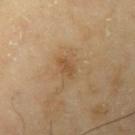Captured during whole-body skin photography for melanoma surveillance; the lesion was not biopsied. This image is a 15 mm lesion crop taken from a total-body photograph. This is a cross-polarized tile. On the left upper arm. A male patient approximately 65 years of age. Approximately 2.5 mm at its widest.